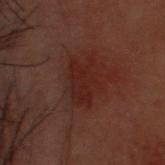Imaged during a routine full-body skin examination; the lesion was not biopsied and no histopathology is available. The lesion is on the head or neck. Longest diameter approximately 4.5 mm. Automated tile analysis of the lesion measured a border-irregularity rating of about 4.5/10, a within-lesion color-variation index near 2/10, and a peripheral color-asymmetry measure near 0.5. The analysis additionally found a classifier nevus-likeness of about 20/100 and a lesion-detection confidence of about 100/100. This is a cross-polarized tile. A region of skin cropped from a whole-body photographic capture, roughly 15 mm wide. A male subject, aged around 30.